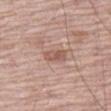Captured during whole-body skin photography for melanoma surveillance; the lesion was not biopsied.
Located on the left thigh.
The patient is a male roughly 70 years of age.
This image is a 15 mm lesion crop taken from a total-body photograph.